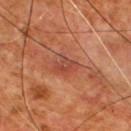Findings:
- image — total-body-photography crop, ~15 mm field of view
- lesion diameter — about 3 mm
- subject — male, aged approximately 55
- anatomic site — the front of the torso
- lighting — cross-polarized illumination